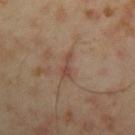Q: Was a biopsy performed?
A: total-body-photography surveillance lesion; no biopsy
Q: Patient demographics?
A: male, roughly 45 years of age
Q: Illumination type?
A: cross-polarized illumination
Q: Where on the body is the lesion?
A: the left upper arm
Q: What is the imaging modality?
A: 15 mm crop, total-body photography
Q: How large is the lesion?
A: ≈3.5 mm
Q: Automated lesion metrics?
A: an area of roughly 3.5 mm² and an eccentricity of roughly 0.9; a border-irregularity rating of about 5.5/10; a classifier nevus-likeness of about 0/100 and lesion-presence confidence of about 100/100